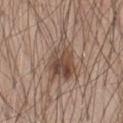| feature | finding |
|---|---|
| notes | imaged on a skin check; not biopsied |
| body site | the chest |
| diameter | about 7 mm |
| subject | male, aged 43 to 47 |
| acquisition | total-body-photography crop, ~15 mm field of view |
| image-analysis metrics | a lesion–skin lightness drop of about 11 and a normalized lesion–skin contrast near 8 |